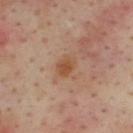This lesion was catalogued during total-body skin photography and was not selected for biopsy. A male patient in their mid- to late 40s. An algorithmic analysis of the crop reported a lesion area of about 4 mm² and an eccentricity of roughly 0.6. And it measured roughly 8 lightness units darker than nearby skin and a lesion-to-skin contrast of about 7.5 (normalized; higher = more distinct). The analysis additionally found a border-irregularity index near 1.5/10, a color-variation rating of about 2/10, and peripheral color asymmetry of about 0.5. The software also gave a classifier nevus-likeness of about 70/100 and a detector confidence of about 100 out of 100 that the crop contains a lesion. A close-up tile cropped from a whole-body skin photograph, about 15 mm across. The tile uses cross-polarized illumination. The lesion is on the upper back.